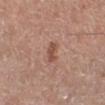Assessment:
Recorded during total-body skin imaging; not selected for excision or biopsy.
Acquisition and patient details:
Located on the right lower leg. The recorded lesion diameter is about 2.5 mm. This is a white-light tile. A close-up tile cropped from a whole-body skin photograph, about 15 mm across. The total-body-photography lesion software estimated a lesion color around L≈51 a*≈23 b*≈28 in CIELAB and about 9 CIELAB-L* units darker than the surrounding skin. The subject is a male aged 58 to 62.